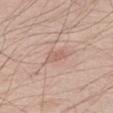No biopsy was performed on this lesion — it was imaged during a full skin examination and was not determined to be concerning. On the right thigh. This image is a 15 mm lesion crop taken from a total-body photograph. The patient is a male in their 60s.